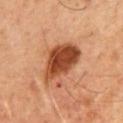The lesion was photographed on a routine skin check and not biopsied; there is no pathology result.
Imaged with cross-polarized lighting.
A male subject, aged 48 to 52.
About 6 mm across.
The lesion is located on the chest.
A 15 mm close-up extracted from a 3D total-body photography capture.
An algorithmic analysis of the crop reported border irregularity of about 2.5 on a 0–10 scale, a within-lesion color-variation index near 5.5/10, and radial color variation of about 2.